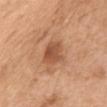| field | value |
|---|---|
| follow-up | imaged on a skin check; not biopsied |
| subject | female, about 55 years old |
| image | ~15 mm tile from a whole-body skin photo |
| lesion size | ≈3 mm |
| location | the chest |
| lighting | white-light illumination |